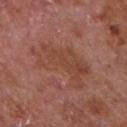The subject is a male about 65 years old.
Located on the front of the torso.
Measured at roughly 7.5 mm in maximum diameter.
Imaged with white-light lighting.
A region of skin cropped from a whole-body photographic capture, roughly 15 mm wide.